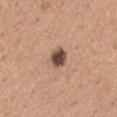Imaged during a routine full-body skin examination; the lesion was not biopsied and no histopathology is available.
The subject is a male in their mid- to late 40s.
Imaged with white-light lighting.
The lesion-visualizer software estimated a mean CIELAB color near L≈48 a*≈18 b*≈25 and about 17 CIELAB-L* units darker than the surrounding skin. The analysis additionally found lesion-presence confidence of about 100/100.
The lesion's longest dimension is about 3 mm.
The lesion is on the right upper arm.
A lesion tile, about 15 mm wide, cut from a 3D total-body photograph.